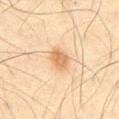{
  "biopsy_status": "not biopsied; imaged during a skin examination",
  "patient": {
    "sex": "male",
    "age_approx": 65
  },
  "image": {
    "source": "total-body photography crop",
    "field_of_view_mm": 15
  },
  "site": "abdomen"
}